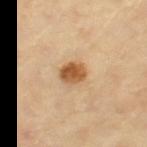Findings:
• biopsy status · no biopsy performed (imaged during a skin exam)
• image source · total-body-photography crop, ~15 mm field of view
• lesion diameter · ~3 mm (longest diameter)
• patient · female, aged around 70
• location · the left thigh